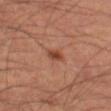biopsy status=no biopsy performed (imaged during a skin exam).